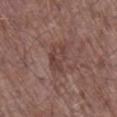Q: Was this lesion biopsied?
A: no biopsy performed (imaged during a skin exam)
Q: What lighting was used for the tile?
A: white-light illumination
Q: Automated lesion metrics?
A: a lesion area of about 6 mm², an eccentricity of roughly 0.8, and a shape-asymmetry score of about 0.4 (0 = symmetric); a border-irregularity index near 5/10, internal color variation of about 3 on a 0–10 scale, and radial color variation of about 1; lesion-presence confidence of about 95/100
Q: Who is the patient?
A: male, aged 78 to 82
Q: What is the imaging modality?
A: total-body-photography crop, ~15 mm field of view
Q: How large is the lesion?
A: ≈3.5 mm
Q: Where on the body is the lesion?
A: the right lower leg